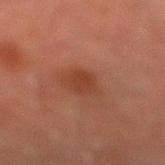A male patient, aged around 70.
Imaged with cross-polarized lighting.
The lesion is located on the left lower leg.
The lesion's longest dimension is about 2.5 mm.
A lesion tile, about 15 mm wide, cut from a 3D total-body photograph.